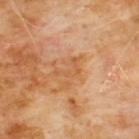Assessment:
Recorded during total-body skin imaging; not selected for excision or biopsy.
Background:
A region of skin cropped from a whole-body photographic capture, roughly 15 mm wide. A male patient approximately 60 years of age. Measured at roughly 3.5 mm in maximum diameter. On the chest. The total-body-photography lesion software estimated a lesion area of about 4 mm², an outline eccentricity of about 0.8 (0 = round, 1 = elongated), and a symmetry-axis asymmetry near 0.75. And it measured border irregularity of about 8 on a 0–10 scale, internal color variation of about 0 on a 0–10 scale, and radial color variation of about 0. And it measured a nevus-likeness score of about 0/100 and lesion-presence confidence of about 100/100.